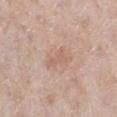workup: total-body-photography surveillance lesion; no biopsy
image source: ~15 mm crop, total-body skin-cancer survey
subject: female, about 60 years old
location: the right lower leg
lighting: white-light
lesion diameter: ~3 mm (longest diameter)